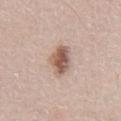Assessment: The lesion was tiled from a total-body skin photograph and was not biopsied. Context: The patient is a male aged around 65. The tile uses white-light illumination. A close-up tile cropped from a whole-body skin photograph, about 15 mm across. Approximately 4 mm at its widest. On the abdomen. An algorithmic analysis of the crop reported a footprint of about 8 mm², an outline eccentricity of about 0.75 (0 = round, 1 = elongated), and a symmetry-axis asymmetry near 0.2. The analysis additionally found a mean CIELAB color near L≈57 a*≈18 b*≈26, roughly 14 lightness units darker than nearby skin, and a lesion-to-skin contrast of about 9.5 (normalized; higher = more distinct). The analysis additionally found a border-irregularity rating of about 2/10, internal color variation of about 6 on a 0–10 scale, and radial color variation of about 2.